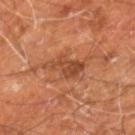Q: Was this lesion biopsied?
A: total-body-photography surveillance lesion; no biopsy